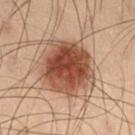notes = no biopsy performed (imaged during a skin exam)
location = the right thigh
subject = male, in their mid- to late 50s
tile lighting = cross-polarized illumination
diameter = about 6.5 mm
image source = ~15 mm crop, total-body skin-cancer survey